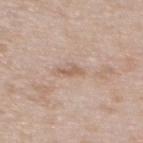Part of a total-body skin-imaging series; this lesion was reviewed on a skin check and was not flagged for biopsy.
The subject is a female aged 28–32.
Located on the upper back.
This image is a 15 mm lesion crop taken from a total-body photograph.